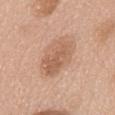  biopsy_status: not biopsied; imaged during a skin examination
  lighting: white-light
  lesion_size:
    long_diameter_mm_approx: 6.0
  image:
    source: total-body photography crop
    field_of_view_mm: 15
  site: abdomen
  automated_metrics:
    cielab_L: 60
    cielab_a: 20
    cielab_b: 31
    vs_skin_darker_L: 9.0
    vs_skin_contrast_norm: 6.0
    border_irregularity_0_10: 2.0
    peripheral_color_asymmetry: 1.5
  patient:
    sex: female
    age_approx: 60